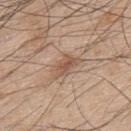Recorded during total-body skin imaging; not selected for excision or biopsy. This is a white-light tile. A roughly 15 mm field-of-view crop from a total-body skin photograph. A male patient, in their mid- to late 40s. On the upper back.